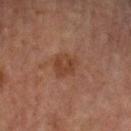{"image": {"source": "total-body photography crop", "field_of_view_mm": 15}, "patient": {"sex": "female", "age_approx": 60}, "site": "right lower leg", "lighting": "cross-polarized", "lesion_size": {"long_diameter_mm_approx": 3.0}, "automated_metrics": {"area_mm2_approx": 7.0, "eccentricity": 0.45, "shape_asymmetry": 0.25, "cielab_L": 36, "cielab_a": 20, "cielab_b": 27, "vs_skin_darker_L": 6.0, "vs_skin_contrast_norm": 6.5, "border_irregularity_0_10": 2.5, "peripheral_color_asymmetry": 1.0}}